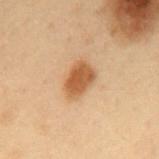Assessment: No biopsy was performed on this lesion — it was imaged during a full skin examination and was not determined to be concerning. Clinical summary: The lesion is located on the mid back. The tile uses cross-polarized illumination. The recorded lesion diameter is about 4 mm. An algorithmic analysis of the crop reported a shape eccentricity near 0.75. The subject is a male in their mid-50s. A close-up tile cropped from a whole-body skin photograph, about 15 mm across.